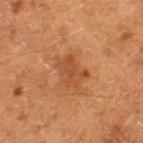Clinical impression:
Imaged during a routine full-body skin examination; the lesion was not biopsied and no histopathology is available.
Image and clinical context:
The lesion-visualizer software estimated a nevus-likeness score of about 20/100 and lesion-presence confidence of about 100/100. A lesion tile, about 15 mm wide, cut from a 3D total-body photograph. On the left thigh. A female subject roughly 50 years of age. About 3.5 mm across. This is a cross-polarized tile.